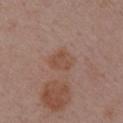automated lesion analysis = an area of roughly 6.5 mm², a shape eccentricity near 0.6, and a symmetry-axis asymmetry near 0.3; border irregularity of about 2.5 on a 0–10 scale, a within-lesion color-variation index near 2/10, and radial color variation of about 0.5
subject = female, in their mid- to late 50s
lesion size = ~3.5 mm (longest diameter)
location = the right upper arm
lighting = white-light
imaging modality = total-body-photography crop, ~15 mm field of view